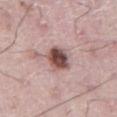Impression: No biopsy was performed on this lesion — it was imaged during a full skin examination and was not determined to be concerning.